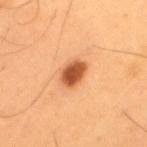The lesion was tiled from a total-body skin photograph and was not biopsied.